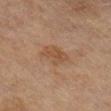Notes:
• follow-up · catalogued during a skin exam; not biopsied
• imaging modality · total-body-photography crop, ~15 mm field of view
• lesion size · about 3.5 mm
• patient · female, in their mid-50s
• location · the right lower leg